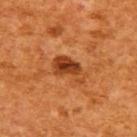lesion size = about 4 mm | anatomic site = the upper back | tile lighting = cross-polarized | image source = ~15 mm crop, total-body skin-cancer survey | patient = female, in their mid- to late 50s.